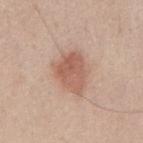follow-up: imaged on a skin check; not biopsied | imaging modality: ~15 mm tile from a whole-body skin photo | body site: the mid back | lesion size: ~5 mm (longest diameter) | patient: male, approximately 50 years of age | lighting: white-light | automated lesion analysis: a lesion area of about 12 mm², an eccentricity of roughly 0.7, and two-axis asymmetry of about 0.3; an automated nevus-likeness rating near 85 out of 100.